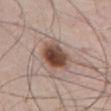Impression:
Captured during whole-body skin photography for melanoma surveillance; the lesion was not biopsied.
Image and clinical context:
Approximately 4.5 mm at its widest. The subject is a male aged approximately 55. A roughly 15 mm field-of-view crop from a total-body skin photograph. Automated tile analysis of the lesion measured a footprint of about 12 mm². And it measured border irregularity of about 2 on a 0–10 scale, a color-variation rating of about 8.5/10, and radial color variation of about 2.5. The lesion is on the abdomen.